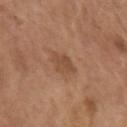<record>
  <biopsy_status>not biopsied; imaged during a skin examination</biopsy_status>
  <lighting>white-light</lighting>
  <automated_metrics>
    <cielab_L>48</cielab_L>
    <cielab_a>21</cielab_a>
    <cielab_b>32</cielab_b>
    <vs_skin_contrast_norm>6.0</vs_skin_contrast_norm>
    <nevus_likeness_0_100>10</nevus_likeness_0_100>
    <lesion_detection_confidence_0_100>100</lesion_detection_confidence_0_100>
  </automated_metrics>
  <lesion_size>
    <long_diameter_mm_approx>3.0</long_diameter_mm_approx>
  </lesion_size>
  <image>
    <source>total-body photography crop</source>
    <field_of_view_mm>15</field_of_view_mm>
  </image>
  <site>left upper arm</site>
  <patient>
    <sex>female</sex>
    <age_approx>65</age_approx>
  </patient>
</record>